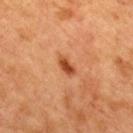  biopsy_status: not biopsied; imaged during a skin examination
  site: mid back
  image:
    source: total-body photography crop
    field_of_view_mm: 15
  lesion_size:
    long_diameter_mm_approx: 2.5
  automated_metrics:
    shape_asymmetry: 0.2
    border_irregularity_0_10: 2.0
    color_variation_0_10: 2.0
  patient:
    sex: female
    age_approx: 40
  lighting: cross-polarized